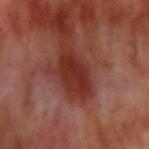Image and clinical context:
The subject is a male aged 68–72. A 15 mm crop from a total-body photograph taken for skin-cancer surveillance. The lesion is located on the right upper arm. An algorithmic analysis of the crop reported a within-lesion color-variation index near 3.5/10.